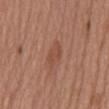– follow-up · total-body-photography surveillance lesion; no biopsy
– acquisition · ~15 mm crop, total-body skin-cancer survey
– lesion diameter · ≈3.5 mm
– subject · male, about 65 years old
– tile lighting · white-light illumination
– body site · the abdomen